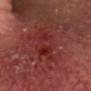{"biopsy_status": "not biopsied; imaged during a skin examination", "site": "head or neck", "patient": {"sex": "male", "age_approx": 65}, "lighting": "cross-polarized", "lesion_size": {"long_diameter_mm_approx": 7.0}, "automated_metrics": {"vs_skin_contrast_norm": 6.0, "border_irregularity_0_10": 6.5, "peripheral_color_asymmetry": 1.5, "nevus_likeness_0_100": 0, "lesion_detection_confidence_0_100": 80}, "image": {"source": "total-body photography crop", "field_of_view_mm": 15}}